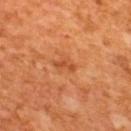- workup: imaged on a skin check; not biopsied
- subject: male, aged approximately 65
- lesion size: ~2.5 mm (longest diameter)
- image source: ~15 mm tile from a whole-body skin photo
- location: the upper back
- TBP lesion metrics: an eccentricity of roughly 0.95 and a symmetry-axis asymmetry near 0.4; a lesion–skin lightness drop of about 8 and a normalized border contrast of about 6; border irregularity of about 4.5 on a 0–10 scale and radial color variation of about 0